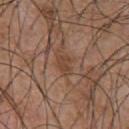<tbp_lesion>
  <biopsy_status>not biopsied; imaged during a skin examination</biopsy_status>
  <site>chest</site>
  <patient>
    <sex>male</sex>
    <age_approx>55</age_approx>
  </patient>
  <image>
    <source>total-body photography crop</source>
    <field_of_view_mm>15</field_of_view_mm>
  </image>
</tbp_lesion>